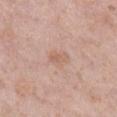Recorded during total-body skin imaging; not selected for excision or biopsy. Automated tile analysis of the lesion measured an eccentricity of roughly 0.8 and a shape-asymmetry score of about 0.25 (0 = symmetric). The software also gave roughly 7 lightness units darker than nearby skin. And it measured a peripheral color-asymmetry measure near 1. The lesion is on the leg. The tile uses white-light illumination. Approximately 3 mm at its widest. A female patient aged 48 to 52. This image is a 15 mm lesion crop taken from a total-body photograph.